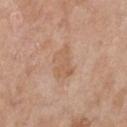{
  "biopsy_status": "not biopsied; imaged during a skin examination",
  "image": {
    "source": "total-body photography crop",
    "field_of_view_mm": 15
  },
  "lesion_size": {
    "long_diameter_mm_approx": 4.0
  },
  "patient": {
    "sex": "female",
    "age_approx": 60
  },
  "site": "chest"
}